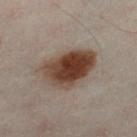Recorded during total-body skin imaging; not selected for excision or biopsy. A male patient in their 50s. Located on the left leg. An algorithmic analysis of the crop reported a lesion area of about 19 mm² and a shape eccentricity near 0.7. It also reported a lesion color around L≈32 a*≈14 b*≈21 in CIELAB. The software also gave a border-irregularity index near 2/10 and internal color variation of about 6 on a 0–10 scale. The analysis additionally found an automated nevus-likeness rating near 100 out of 100. The recorded lesion diameter is about 6 mm. A 15 mm close-up extracted from a 3D total-body photography capture.